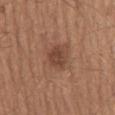Clinical impression: Recorded during total-body skin imaging; not selected for excision or biopsy. Context: Imaged with white-light lighting. Located on the lower back. Automated tile analysis of the lesion measured a lesion area of about 6 mm², a shape eccentricity near 0.45, and a shape-asymmetry score of about 0.3 (0 = symmetric). The software also gave a lesion color around L≈43 a*≈21 b*≈27 in CIELAB, about 9 CIELAB-L* units darker than the surrounding skin, and a normalized lesion–skin contrast near 7. And it measured a border-irregularity index near 2.5/10 and a within-lesion color-variation index near 2/10. It also reported a nevus-likeness score of about 50/100 and a detector confidence of about 100 out of 100 that the crop contains a lesion. A close-up tile cropped from a whole-body skin photograph, about 15 mm across. A male patient aged around 65.